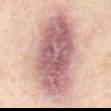  biopsy_status: not biopsied; imaged during a skin examination
  automated_metrics:
    area_mm2_approx: 65.0
    shape_asymmetry: 0.15
    cielab_L: 61
    cielab_a: 20
    cielab_b: 20
    vs_skin_darker_L: 16.0
    vs_skin_contrast_norm: 10.5
    border_irregularity_0_10: 2.5
    color_variation_0_10: 8.5
    peripheral_color_asymmetry: 3.0
    nevus_likeness_0_100: 10
  image:
    source: total-body photography crop
    field_of_view_mm: 15
  patient:
    sex: female
    age_approx: 50
  site: abdomen